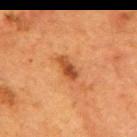Captured during whole-body skin photography for melanoma surveillance; the lesion was not biopsied. Measured at roughly 3.5 mm in maximum diameter. The tile uses cross-polarized illumination. On the mid back. A female patient roughly 50 years of age. Cropped from a total-body skin-imaging series; the visible field is about 15 mm.